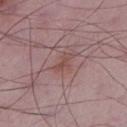  biopsy_status: not biopsied; imaged during a skin examination
  patient:
    sex: male
    age_approx: 50
  image:
    source: total-body photography crop
    field_of_view_mm: 15
  lighting: white-light
  lesion_size:
    long_diameter_mm_approx: 3.0
  site: left lower leg